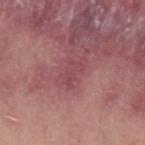<case>
<biopsy_status>not biopsied; imaged during a skin examination</biopsy_status>
<lesion_size>
  <long_diameter_mm_approx>3.0</long_diameter_mm_approx>
</lesion_size>
<site>left upper arm</site>
<image>
  <source>total-body photography crop</source>
  <field_of_view_mm>15</field_of_view_mm>
</image>
<patient>
  <sex>male</sex>
  <age_approx>30</age_approx>
</patient>
</case>